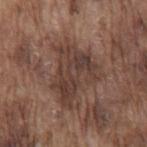biopsy status=imaged on a skin check; not biopsied
imaging modality=~15 mm crop, total-body skin-cancer survey
lesion size=about 6.5 mm
location=the mid back
subject=male, approximately 75 years of age
lighting=white-light illumination